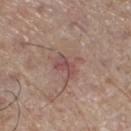Case summary:
* follow-up · imaged on a skin check; not biopsied
* lighting · white-light
* anatomic site · the left lower leg
* patient · male, aged around 70
* acquisition · ~15 mm crop, total-body skin-cancer survey